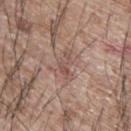image:
  source: total-body photography crop
  field_of_view_mm: 15
site: upper back
patient:
  sex: male
  age_approx: 65
automated_metrics:
  area_mm2_approx: 3.5
  eccentricity: 0.95
  shape_asymmetry: 0.55
  vs_skin_darker_L: 8.0
  vs_skin_contrast_norm: 6.0